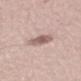Recorded during total-body skin imaging; not selected for excision or biopsy. About 3.5 mm across. A close-up tile cropped from a whole-body skin photograph, about 15 mm across. From the right upper arm. A male patient, aged approximately 60. This is a white-light tile.